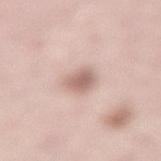Imaged during a routine full-body skin examination; the lesion was not biopsied and no histopathology is available. The lesion is located on the lower back. Automated tile analysis of the lesion measured a within-lesion color-variation index near 2.5/10 and radial color variation of about 0.5. The software also gave a lesion-detection confidence of about 100/100. A close-up tile cropped from a whole-body skin photograph, about 15 mm across. A female subject, approximately 50 years of age.